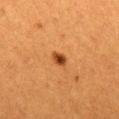Clinical impression: Imaged during a routine full-body skin examination; the lesion was not biopsied and no histopathology is available. Background: On the mid back. This image is a 15 mm lesion crop taken from a total-body photograph. The subject is a female roughly 55 years of age. Imaged with cross-polarized lighting.